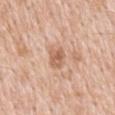The lesion was tiled from a total-body skin photograph and was not biopsied. Measured at roughly 3 mm in maximum diameter. From the mid back. Imaged with white-light lighting. A male patient, about 50 years old. A 15 mm close-up tile from a total-body photography series done for melanoma screening.